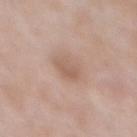Part of a total-body skin-imaging series; this lesion was reviewed on a skin check and was not flagged for biopsy.
A male patient approximately 55 years of age.
A lesion tile, about 15 mm wide, cut from a 3D total-body photograph.
Longest diameter approximately 3.5 mm.
On the front of the torso.
Imaged with white-light lighting.
Automated image analysis of the tile measured a lesion–skin lightness drop of about 8. And it measured a nevus-likeness score of about 60/100 and lesion-presence confidence of about 100/100.